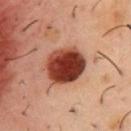No biopsy was performed on this lesion — it was imaged during a full skin examination and was not determined to be concerning. Approximately 5 mm at its widest. A male subject aged around 50. From the front of the torso. Automated image analysis of the tile measured an area of roughly 17 mm². The software also gave a within-lesion color-variation index near 7/10. And it measured a classifier nevus-likeness of about 100/100 and a lesion-detection confidence of about 100/100. A close-up tile cropped from a whole-body skin photograph, about 15 mm across.